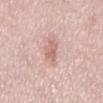The lesion was photographed on a routine skin check and not biopsied; there is no pathology result. The lesion's longest dimension is about 3.5 mm. The patient is a male approximately 50 years of age. Automated image analysis of the tile measured a nevus-likeness score of about 0/100 and lesion-presence confidence of about 100/100. A 15 mm crop from a total-body photograph taken for skin-cancer surveillance. On the front of the torso. The tile uses white-light illumination.